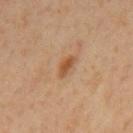Image and clinical context:
A male subject, aged approximately 60. A region of skin cropped from a whole-body photographic capture, roughly 15 mm wide. On the mid back.